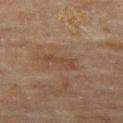Impression: Part of a total-body skin-imaging series; this lesion was reviewed on a skin check and was not flagged for biopsy.